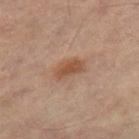Impression:
The lesion was tiled from a total-body skin photograph and was not biopsied.
Acquisition and patient details:
From the leg. The tile uses cross-polarized illumination. The patient is a female roughly 80 years of age. A 15 mm close-up tile from a total-body photography series done for melanoma screening.